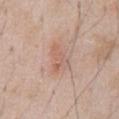Part of a total-body skin-imaging series; this lesion was reviewed on a skin check and was not flagged for biopsy.
The subject is a male aged around 60.
A 15 mm close-up extracted from a 3D total-body photography capture.
The lesion is on the abdomen.